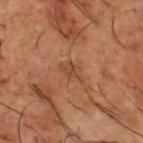Findings:
– biopsy status: imaged on a skin check; not biopsied
– lighting: cross-polarized illumination
– anatomic site: the right thigh
– automated lesion analysis: an area of roughly 4 mm²; a lesion color around L≈46 a*≈23 b*≈32 in CIELAB, roughly 7 lightness units darker than nearby skin, and a normalized border contrast of about 5.5; a border-irregularity index near 4/10 and peripheral color asymmetry of about 1.5
– image source: 15 mm crop, total-body photography
– diameter: about 3 mm
– patient: male, roughly 65 years of age